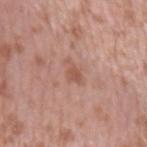Clinical impression: Imaged during a routine full-body skin examination; the lesion was not biopsied and no histopathology is available. Background: Cropped from a whole-body photographic skin survey; the tile spans about 15 mm. The lesion is located on the arm. Longest diameter approximately 3 mm. The tile uses white-light illumination. The subject is a male in their mid- to late 50s.